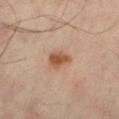Part of a total-body skin-imaging series; this lesion was reviewed on a skin check and was not flagged for biopsy. The lesion is on the left lower leg. The subject is a male aged around 65. This image is a 15 mm lesion crop taken from a total-body photograph.